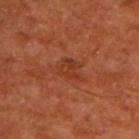Imaged during a routine full-body skin examination; the lesion was not biopsied and no histopathology is available. Measured at roughly 3 mm in maximum diameter. Located on the upper back. A male patient aged 63–67. The total-body-photography lesion software estimated roughly 6 lightness units darker than nearby skin and a normalized border contrast of about 6. It also reported a border-irregularity index near 4.5/10 and a peripheral color-asymmetry measure near 1. The software also gave a nevus-likeness score of about 0/100 and a lesion-detection confidence of about 100/100. A lesion tile, about 15 mm wide, cut from a 3D total-body photograph.